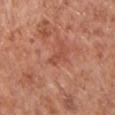{
  "biopsy_status": "not biopsied; imaged during a skin examination",
  "image": {
    "source": "total-body photography crop",
    "field_of_view_mm": 15
  },
  "site": "chest",
  "lesion_size": {
    "long_diameter_mm_approx": 2.5
  },
  "patient": {
    "sex": "male",
    "age_approx": 65
  },
  "automated_metrics": {
    "area_mm2_approx": 2.5,
    "eccentricity": 0.9,
    "nevus_likeness_0_100": 0,
    "lesion_detection_confidence_0_100": 100
  }
}